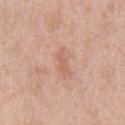Imaged during a routine full-body skin examination; the lesion was not biopsied and no histopathology is available. The recorded lesion diameter is about 2.5 mm. The subject is a male aged approximately 40. On the upper back. Cropped from a total-body skin-imaging series; the visible field is about 15 mm. Captured under white-light illumination.